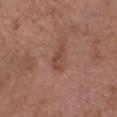Q: Is there a histopathology result?
A: catalogued during a skin exam; not biopsied
Q: Patient demographics?
A: male, aged approximately 50
Q: Where on the body is the lesion?
A: the head or neck
Q: What is the imaging modality?
A: total-body-photography crop, ~15 mm field of view